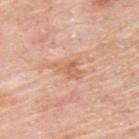workup = no biopsy performed (imaged during a skin exam)
subject = male, aged around 75
acquisition = 15 mm crop, total-body photography
lighting = white-light
automated lesion analysis = a lesion area of about 4.5 mm²; a lesion color around L≈63 a*≈23 b*≈36 in CIELAB, about 8 CIELAB-L* units darker than the surrounding skin, and a normalized border contrast of about 6
anatomic site = the upper back
size = ~3.5 mm (longest diameter)